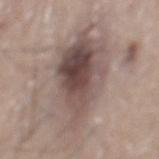The lesion was photographed on a routine skin check and not biopsied; there is no pathology result.
A male patient approximately 75 years of age.
Captured under white-light illumination.
Measured at roughly 10.5 mm in maximum diameter.
The lesion is located on the mid back.
A 15 mm close-up tile from a total-body photography series done for melanoma screening.